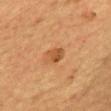Captured during whole-body skin photography for melanoma surveillance; the lesion was not biopsied.
The patient is a male aged around 60.
A 15 mm crop from a total-body photograph taken for skin-cancer surveillance.
The lesion-visualizer software estimated border irregularity of about 2 on a 0–10 scale, internal color variation of about 2.5 on a 0–10 scale, and a peripheral color-asymmetry measure near 1. The analysis additionally found a classifier nevus-likeness of about 80/100.